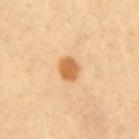This lesion was catalogued during total-body skin photography and was not selected for biopsy. The lesion is located on the mid back. Approximately 2.5 mm at its widest. The patient is a male aged 38–42. A lesion tile, about 15 mm wide, cut from a 3D total-body photograph.